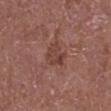Recorded during total-body skin imaging; not selected for excision or biopsy.
The recorded lesion diameter is about 3 mm.
A close-up tile cropped from a whole-body skin photograph, about 15 mm across.
The lesion is on the leg.
The patient is a male in their mid- to late 70s.